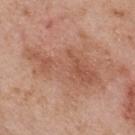biopsy status: catalogued during a skin exam; not biopsied | diameter: ~9.5 mm (longest diameter) | subject: male, in their mid-50s | image source: total-body-photography crop, ~15 mm field of view | anatomic site: the upper back | image-analysis metrics: a footprint of about 25 mm², an eccentricity of roughly 0.9, and a shape-asymmetry score of about 0.45 (0 = symmetric); a mean CIELAB color near L≈55 a*≈22 b*≈31, a lesion–skin lightness drop of about 8, and a lesion-to-skin contrast of about 6 (normalized; higher = more distinct).